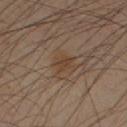notes = total-body-photography surveillance lesion; no biopsy | automated lesion analysis = about 6 CIELAB-L* units darker than the surrounding skin and a normalized lesion–skin contrast near 6; border irregularity of about 3 on a 0–10 scale, a color-variation rating of about 1.5/10, and radial color variation of about 0.5 | body site = the right upper arm | illumination = cross-polarized | patient = male, aged 38–42 | diameter = ≈2.5 mm | acquisition = total-body-photography crop, ~15 mm field of view.